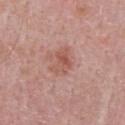The lesion was tiled from a total-body skin photograph and was not biopsied. Approximately 3 mm at its widest. An algorithmic analysis of the crop reported a border-irregularity rating of about 4.5/10, a within-lesion color-variation index near 4/10, and a peripheral color-asymmetry measure near 1.5. It also reported a nevus-likeness score of about 0/100. A roughly 15 mm field-of-view crop from a total-body skin photograph. On the front of the torso. The subject is a male about 50 years old. This is a white-light tile.